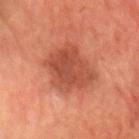Assessment:
This lesion was catalogued during total-body skin photography and was not selected for biopsy.
Context:
Longest diameter approximately 5.5 mm. The total-body-photography lesion software estimated a lesion–skin lightness drop of about 9 and a lesion-to-skin contrast of about 7 (normalized; higher = more distinct). It also reported a border-irregularity rating of about 2.5/10, a color-variation rating of about 3.5/10, and a peripheral color-asymmetry measure near 1. The software also gave a classifier nevus-likeness of about 5/100 and a lesion-detection confidence of about 100/100. The lesion is located on the head or neck. The tile uses cross-polarized illumination. A region of skin cropped from a whole-body photographic capture, roughly 15 mm wide. A male patient about 55 years old.